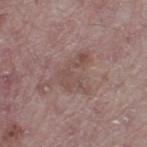A male patient, in their mid-50s. Imaged with white-light lighting. The lesion is located on the leg. An algorithmic analysis of the crop reported an area of roughly 8.5 mm², an eccentricity of roughly 0.75, and a shape-asymmetry score of about 0.6 (0 = symmetric). The software also gave a lesion color around L≈49 a*≈18 b*≈21 in CIELAB and a lesion-to-skin contrast of about 5 (normalized; higher = more distinct). It also reported a border-irregularity index near 8.5/10, internal color variation of about 2 on a 0–10 scale, and radial color variation of about 0.5. A 15 mm close-up tile from a total-body photography series done for melanoma screening. Measured at roughly 4.5 mm in maximum diameter.